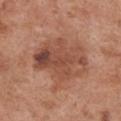Clinical impression:
Imaged during a routine full-body skin examination; the lesion was not biopsied and no histopathology is available.
Background:
Captured under white-light illumination. A female subject, in their mid-60s. Measured at roughly 7 mm in maximum diameter. Cropped from a total-body skin-imaging series; the visible field is about 15 mm. Automated image analysis of the tile measured a mean CIELAB color near L≈49 a*≈23 b*≈30, a lesion–skin lightness drop of about 10, and a normalized border contrast of about 7. It also reported a border-irregularity rating of about 3.5/10 and a color-variation rating of about 7.5/10. From the chest.